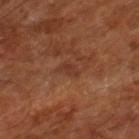{"image": {"source": "total-body photography crop", "field_of_view_mm": 15}, "patient": {"age_approx": 65}, "site": "leg"}